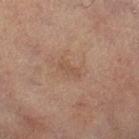Imaged during a routine full-body skin examination; the lesion was not biopsied and no histopathology is available.